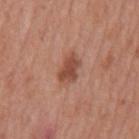{"biopsy_status": "not biopsied; imaged during a skin examination", "site": "back", "automated_metrics": {"area_mm2_approx": 6.5, "eccentricity": 0.8, "shape_asymmetry": 0.3, "border_irregularity_0_10": 3.0, "color_variation_0_10": 2.0, "peripheral_color_asymmetry": 0.5}, "lesion_size": {"long_diameter_mm_approx": 3.5}, "patient": {"sex": "male", "age_approx": 70}, "lighting": "white-light", "image": {"source": "total-body photography crop", "field_of_view_mm": 15}}